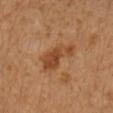Clinical impression:
Captured during whole-body skin photography for melanoma surveillance; the lesion was not biopsied.
Clinical summary:
From the right forearm. A lesion tile, about 15 mm wide, cut from a 3D total-body photograph. The subject is a female in their 40s.